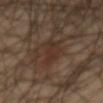{"biopsy_status": "not biopsied; imaged during a skin examination", "site": "abdomen", "image": {"source": "total-body photography crop", "field_of_view_mm": 15}, "patient": {"sex": "male", "age_approx": 60}}